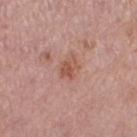Clinical impression:
No biopsy was performed on this lesion — it was imaged during a full skin examination and was not determined to be concerning.
Acquisition and patient details:
Automated tile analysis of the lesion measured a within-lesion color-variation index near 3/10 and peripheral color asymmetry of about 1. A lesion tile, about 15 mm wide, cut from a 3D total-body photograph. Imaged with white-light lighting. A female patient, about 65 years old. From the right thigh. The lesion's longest dimension is about 2.5 mm.